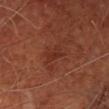Findings:
– workup · no biopsy performed (imaged during a skin exam)
– location · the head or neck
– patient · male, aged 63 to 67
– imaging modality · 15 mm crop, total-body photography
– tile lighting · cross-polarized illumination